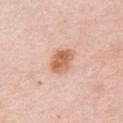{
  "biopsy_status": "not biopsied; imaged during a skin examination",
  "image": {
    "source": "total-body photography crop",
    "field_of_view_mm": 15
  },
  "lesion_size": {
    "long_diameter_mm_approx": 3.5
  },
  "automated_metrics": {
    "area_mm2_approx": 8.0,
    "shape_asymmetry": 0.15,
    "cielab_L": 64,
    "cielab_a": 23,
    "cielab_b": 33,
    "vs_skin_darker_L": 12.0,
    "vs_skin_contrast_norm": 8.5,
    "border_irregularity_0_10": 1.0,
    "peripheral_color_asymmetry": 1.5,
    "nevus_likeness_0_100": 100,
    "lesion_detection_confidence_0_100": 100
  },
  "patient": {
    "sex": "female",
    "age_approx": 65
  },
  "site": "chest",
  "lighting": "white-light"
}